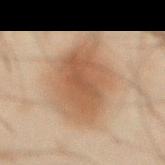| key | value |
|---|---|
| biopsy status | total-body-photography surveillance lesion; no biopsy |
| acquisition | ~15 mm tile from a whole-body skin photo |
| location | the abdomen |
| illumination | cross-polarized |
| subject | male, aged 43 to 47 |